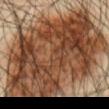<lesion>
<biopsy_status>not biopsied; imaged during a skin examination</biopsy_status>
<automated_metrics>
  <area_mm2_approx>130.0</area_mm2_approx>
  <eccentricity>0.75</eccentricity>
  <shape_asymmetry>0.2</shape_asymmetry>
  <cielab_L>42</cielab_L>
  <cielab_a>19</cielab_a>
  <cielab_b>30</cielab_b>
  <vs_skin_darker_L>17.0</vs_skin_darker_L>
  <vs_skin_contrast_norm>12.5</vs_skin_contrast_norm>
  <border_irregularity_0_10>4.0</border_irregularity_0_10>
  <color_variation_0_10>9.0</color_variation_0_10>
  <peripheral_color_asymmetry>3.5</peripheral_color_asymmetry>
  <lesion_detection_confidence_0_100>95</lesion_detection_confidence_0_100>
</automated_metrics>
<site>abdomen</site>
<patient>
  <sex>male</sex>
  <age_approx>45</age_approx>
</patient>
<image>
  <source>total-body photography crop</source>
  <field_of_view_mm>15</field_of_view_mm>
</image>
</lesion>